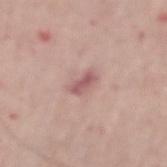Captured during whole-body skin photography for melanoma surveillance; the lesion was not biopsied.
Approximately 3 mm at its widest.
A 15 mm close-up tile from a total-body photography series done for melanoma screening.
A male patient aged approximately 65.
The lesion is located on the right forearm.
Imaged with white-light lighting.